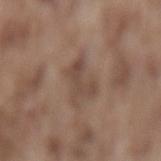Image and clinical context: The lesion is on the lower back. The patient is a male aged 73–77. Longest diameter approximately 4 mm. Cropped from a total-body skin-imaging series; the visible field is about 15 mm.